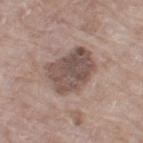Q: Was this lesion biopsied?
A: total-body-photography surveillance lesion; no biopsy
Q: Patient demographics?
A: female, about 75 years old
Q: How was this image acquired?
A: total-body-photography crop, ~15 mm field of view
Q: Where on the body is the lesion?
A: the left thigh